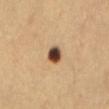location: the abdomen | acquisition: ~15 mm crop, total-body skin-cancer survey | patient: female, approximately 30 years of age.